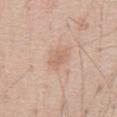notes: total-body-photography surveillance lesion; no biopsy.